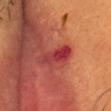{"biopsy_status": "not biopsied; imaged during a skin examination", "site": "head or neck", "lesion_size": {"long_diameter_mm_approx": 4.5}, "lighting": "cross-polarized", "patient": {"sex": "male", "age_approx": 60}, "image": {"source": "total-body photography crop", "field_of_view_mm": 15}}